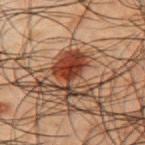Q: Was this lesion biopsied?
A: imaged on a skin check; not biopsied
Q: Automated lesion metrics?
A: a footprint of about 12 mm², a shape eccentricity near 0.85, and a shape-asymmetry score of about 0.6 (0 = symmetric); an automated nevus-likeness rating near 100 out of 100 and a detector confidence of about 100 out of 100 that the crop contains a lesion
Q: Who is the patient?
A: male, roughly 50 years of age
Q: How was this image acquired?
A: 15 mm crop, total-body photography
Q: How was the tile lit?
A: cross-polarized
Q: Lesion location?
A: the left upper arm
Q: What is the lesion's diameter?
A: ≈6 mm